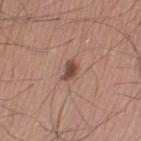| feature | finding |
|---|---|
| workup | total-body-photography surveillance lesion; no biopsy |
| automated lesion analysis | a footprint of about 3.5 mm², a shape eccentricity near 0.75, and a shape-asymmetry score of about 0.35 (0 = symmetric); border irregularity of about 3 on a 0–10 scale, a within-lesion color-variation index near 3/10, and radial color variation of about 1; a detector confidence of about 100 out of 100 that the crop contains a lesion |
| site | the left thigh |
| illumination | white-light |
| lesion diameter | ≈2.5 mm |
| subject | male, aged approximately 35 |
| imaging modality | 15 mm crop, total-body photography |